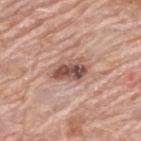The lesion was photographed on a routine skin check and not biopsied; there is no pathology result.
Cropped from a whole-body photographic skin survey; the tile spans about 15 mm.
The lesion is on the upper back.
A male subject, aged 78–82.
Longest diameter approximately 4.5 mm.
The tile uses white-light illumination.